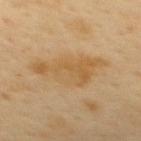{"biopsy_status": "not biopsied; imaged during a skin examination", "image": {"source": "total-body photography crop", "field_of_view_mm": 15}, "patient": {"sex": "female", "age_approx": 50}, "site": "upper back"}